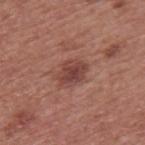Background:
The tile uses white-light illumination. The lesion's longest dimension is about 3.5 mm. A male subject, in their mid-70s. From the upper back. A 15 mm crop from a total-body photograph taken for skin-cancer surveillance.